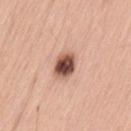Impression: Captured during whole-body skin photography for melanoma surveillance; the lesion was not biopsied. Clinical summary: A male subject aged 58–62. The total-body-photography lesion software estimated a border-irregularity index near 1.5/10, a color-variation rating of about 8/10, and peripheral color asymmetry of about 2. On the back. Measured at roughly 3.5 mm in maximum diameter. Cropped from a total-body skin-imaging series; the visible field is about 15 mm.